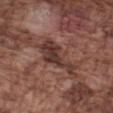Clinical impression:
Part of a total-body skin-imaging series; this lesion was reviewed on a skin check and was not flagged for biopsy.
Clinical summary:
A male subject, aged around 75. The total-body-photography lesion software estimated a lesion area of about 12 mm² and a symmetry-axis asymmetry near 0.5. It also reported a mean CIELAB color near L≈36 a*≈20 b*≈22, roughly 11 lightness units darker than nearby skin, and a lesion-to-skin contrast of about 9.5 (normalized; higher = more distinct). Captured under white-light illumination. The lesion is on the left forearm. A 15 mm crop from a total-body photograph taken for skin-cancer surveillance. About 6 mm across.